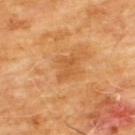Part of a total-body skin-imaging series; this lesion was reviewed on a skin check and was not flagged for biopsy.